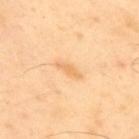The tile uses cross-polarized illumination.
A male patient about 40 years old.
Cropped from a whole-body photographic skin survey; the tile spans about 15 mm.
The lesion-visualizer software estimated a border-irregularity index near 4.5/10, a color-variation rating of about 0/10, and radial color variation of about 0.
The lesion is on the upper back.